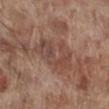Assessment: Recorded during total-body skin imaging; not selected for excision or biopsy. Background: A close-up tile cropped from a whole-body skin photograph, about 15 mm across. From the right lower leg. The subject is a male aged around 70.